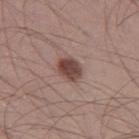No biopsy was performed on this lesion — it was imaged during a full skin examination and was not determined to be concerning. This is a white-light tile. The subject is a male aged 28–32. On the left thigh. About 3 mm across. A lesion tile, about 15 mm wide, cut from a 3D total-body photograph.